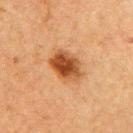Impression:
Imaged during a routine full-body skin examination; the lesion was not biopsied and no histopathology is available.
Image and clinical context:
Captured under cross-polarized illumination. The lesion is located on the right upper arm. Measured at roughly 4 mm in maximum diameter. A female subject, roughly 60 years of age. A lesion tile, about 15 mm wide, cut from a 3D total-body photograph. Automated image analysis of the tile measured a border-irregularity rating of about 2/10, internal color variation of about 6 on a 0–10 scale, and peripheral color asymmetry of about 2.